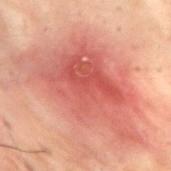Q: Was a biopsy performed?
A: no biopsy performed (imaged during a skin exam)
Q: How large is the lesion?
A: ≈14 mm
Q: Where on the body is the lesion?
A: the back
Q: What is the imaging modality?
A: ~15 mm tile from a whole-body skin photo
Q: Who is the patient?
A: male, in their 30s